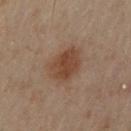| feature | finding |
|---|---|
| workup | imaged on a skin check; not biopsied |
| tile lighting | cross-polarized illumination |
| lesion size | about 4 mm |
| location | the arm |
| subject | male, approximately 50 years of age |
| acquisition | total-body-photography crop, ~15 mm field of view |